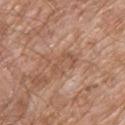Impression:
No biopsy was performed on this lesion — it was imaged during a full skin examination and was not determined to be concerning.
Background:
Automated image analysis of the tile measured a footprint of about 6.5 mm², an outline eccentricity of about 0.8 (0 = round, 1 = elongated), and two-axis asymmetry of about 0.4. The software also gave an average lesion color of about L≈54 a*≈20 b*≈30 (CIELAB), about 7 CIELAB-L* units darker than the surrounding skin, and a lesion-to-skin contrast of about 5 (normalized; higher = more distinct). The tile uses white-light illumination. On the upper back. A male subject about 80 years old. The recorded lesion diameter is about 3.5 mm. A lesion tile, about 15 mm wide, cut from a 3D total-body photograph.